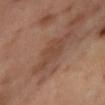notes — imaged on a skin check; not biopsied | lighting — cross-polarized | location — the right thigh | subject — female, about 55 years old | diameter — ≈4.5 mm | acquisition — ~15 mm crop, total-body skin-cancer survey.